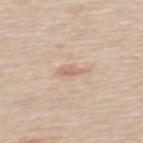<lesion>
<biopsy_status>not biopsied; imaged during a skin examination</biopsy_status>
<site>mid back</site>
<lesion_size>
  <long_diameter_mm_approx>3.0</long_diameter_mm_approx>
</lesion_size>
<image>
  <source>total-body photography crop</source>
  <field_of_view_mm>15</field_of_view_mm>
</image>
<patient>
  <sex>male</sex>
  <age_approx>65</age_approx>
</patient>
<lighting>white-light</lighting>
</lesion>